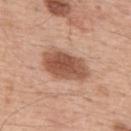  image:
    source: total-body photography crop
    field_of_view_mm: 15
  site: upper back
  patient:
    sex: male
    age_approx: 55